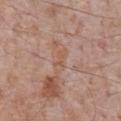Impression: The lesion was tiled from a total-body skin photograph and was not biopsied. Image and clinical context: An algorithmic analysis of the crop reported a shape eccentricity near 0.9 and a shape-asymmetry score of about 0.3 (0 = symmetric). The analysis additionally found border irregularity of about 3.5 on a 0–10 scale and internal color variation of about 2.5 on a 0–10 scale. And it measured a nevus-likeness score of about 0/100 and lesion-presence confidence of about 55/100. Longest diameter approximately 3 mm. A male patient approximately 70 years of age. The lesion is on the chest. A 15 mm close-up tile from a total-body photography series done for melanoma screening. The tile uses white-light illumination.